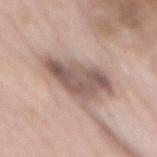Part of a total-body skin-imaging series; this lesion was reviewed on a skin check and was not flagged for biopsy. A female subject aged 63–67. Longest diameter approximately 6.5 mm. Cropped from a total-body skin-imaging series; the visible field is about 15 mm. Located on the mid back. An algorithmic analysis of the crop reported a border-irregularity index near 4.5/10 and peripheral color asymmetry of about 1.5. It also reported a classifier nevus-likeness of about 5/100 and a detector confidence of about 100 out of 100 that the crop contains a lesion.